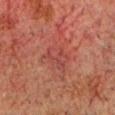Imaged during a routine full-body skin examination; the lesion was not biopsied and no histopathology is available. Captured under cross-polarized illumination. A male subject, aged 58 to 62. A close-up tile cropped from a whole-body skin photograph, about 15 mm across. The lesion is located on the head or neck. The lesion-visualizer software estimated a footprint of about 8 mm², an eccentricity of roughly 0.75, and a symmetry-axis asymmetry near 0.3. And it measured a lesion color around L≈35 a*≈24 b*≈22 in CIELAB and a normalized lesion–skin contrast near 4.5. And it measured a nevus-likeness score of about 0/100. Measured at roughly 4 mm in maximum diameter.